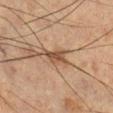  biopsy_status: not biopsied; imaged during a skin examination
  lighting: cross-polarized
  patient:
    sex: male
    age_approx: 60
  site: leg
  image:
    source: total-body photography crop
    field_of_view_mm: 15
  lesion_size:
    long_diameter_mm_approx: 3.5